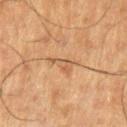No biopsy was performed on this lesion — it was imaged during a full skin examination and was not determined to be concerning. A male patient aged 58–62. An algorithmic analysis of the crop reported a border-irregularity rating of about 6.5/10, a color-variation rating of about 3.5/10, and peripheral color asymmetry of about 1. About 3.5 mm across. A region of skin cropped from a whole-body photographic capture, roughly 15 mm wide. The tile uses cross-polarized illumination. From the left upper arm.